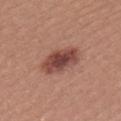Assessment:
No biopsy was performed on this lesion — it was imaged during a full skin examination and was not determined to be concerning.
Image and clinical context:
The lesion's longest dimension is about 5 mm. Located on the upper back. Automated image analysis of the tile measured a lesion-detection confidence of about 100/100. A female subject, roughly 20 years of age. A 15 mm close-up tile from a total-body photography series done for melanoma screening.